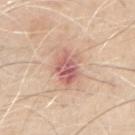Part of a total-body skin-imaging series; this lesion was reviewed on a skin check and was not flagged for biopsy. Captured under white-light illumination. From the right upper arm. About 4.5 mm across. A male patient, aged 63–67. A region of skin cropped from a whole-body photographic capture, roughly 15 mm wide.